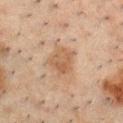follow-up: imaged on a skin check; not biopsied | lesion diameter: ≈4 mm | anatomic site: the chest | acquisition: 15 mm crop, total-body photography | TBP lesion metrics: a footprint of about 9 mm², an outline eccentricity of about 0.55 (0 = round, 1 = elongated), and two-axis asymmetry of about 0.25; a classifier nevus-likeness of about 10/100 and a detector confidence of about 100 out of 100 that the crop contains a lesion | subject: male, aged 48 to 52 | tile lighting: cross-polarized illumination.